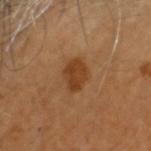Captured during whole-body skin photography for melanoma surveillance; the lesion was not biopsied. On the head or neck. An algorithmic analysis of the crop reported a lesion area of about 6.5 mm², an outline eccentricity of about 0.75 (0 = round, 1 = elongated), and a symmetry-axis asymmetry near 0.2. And it measured a lesion color around L≈40 a*≈23 b*≈36 in CIELAB, roughly 9 lightness units darker than nearby skin, and a lesion-to-skin contrast of about 8 (normalized; higher = more distinct). And it measured border irregularity of about 2 on a 0–10 scale, a within-lesion color-variation index near 2/10, and radial color variation of about 1. Longest diameter approximately 3.5 mm. The subject is a male aged 43–47. A roughly 15 mm field-of-view crop from a total-body skin photograph. The tile uses cross-polarized illumination.